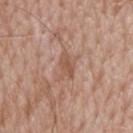This lesion was catalogued during total-body skin photography and was not selected for biopsy. Captured under white-light illumination. A male subject in their mid- to late 60s. The lesion's longest dimension is about 3.5 mm. On the upper back. Cropped from a total-body skin-imaging series; the visible field is about 15 mm. Automated image analysis of the tile measured internal color variation of about 2 on a 0–10 scale and a peripheral color-asymmetry measure near 0.5. The software also gave a detector confidence of about 100 out of 100 that the crop contains a lesion.